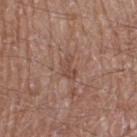{
  "biopsy_status": "not biopsied; imaged during a skin examination",
  "image": {
    "source": "total-body photography crop",
    "field_of_view_mm": 15
  },
  "lighting": "white-light",
  "patient": {
    "sex": "male",
    "age_approx": 65
  },
  "site": "right thigh",
  "lesion_size": {
    "long_diameter_mm_approx": 3.0
  }
}